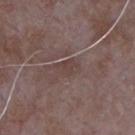Assessment: Part of a total-body skin-imaging series; this lesion was reviewed on a skin check and was not flagged for biopsy. Background: From the chest. The lesion's longest dimension is about 3 mm. Automated image analysis of the tile measured border irregularity of about 3 on a 0–10 scale, a color-variation rating of about 1.5/10, and radial color variation of about 0.5. It also reported a nevus-likeness score of about 0/100. Cropped from a total-body skin-imaging series; the visible field is about 15 mm. A male subject, approximately 65 years of age. Captured under white-light illumination.